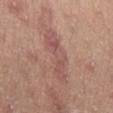Recorded during total-body skin imaging; not selected for excision or biopsy.
The lesion is located on the chest.
The tile uses white-light illumination.
A 15 mm close-up extracted from a 3D total-body photography capture.
The lesion's longest dimension is about 6.5 mm.
An algorithmic analysis of the crop reported a mean CIELAB color near L≈53 a*≈22 b*≈23 and a lesion–skin lightness drop of about 7. The analysis additionally found a nevus-likeness score of about 0/100 and a detector confidence of about 80 out of 100 that the crop contains a lesion.
A female patient in their mid-40s.